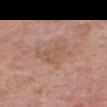This lesion was catalogued during total-body skin photography and was not selected for biopsy.
A 15 mm close-up tile from a total-body photography series done for melanoma screening.
A male patient aged 63–67.
On the chest.